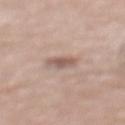workup: no biopsy performed (imaged during a skin exam)
lesion diameter: ≈3 mm
anatomic site: the chest
illumination: white-light illumination
image: 15 mm crop, total-body photography
subject: female, about 65 years old